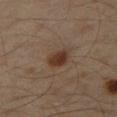From the right thigh. A 15 mm close-up tile from a total-body photography series done for melanoma screening. A male patient about 60 years old.